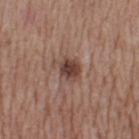This lesion was catalogued during total-body skin photography and was not selected for biopsy. Captured under white-light illumination. Cropped from a total-body skin-imaging series; the visible field is about 15 mm. The recorded lesion diameter is about 2.5 mm. A female subject aged 53 to 57. The lesion is located on the left thigh.